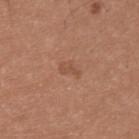No biopsy was performed on this lesion — it was imaged during a full skin examination and was not determined to be concerning.
An algorithmic analysis of the crop reported a footprint of about 3.5 mm², an outline eccentricity of about 0.8 (0 = round, 1 = elongated), and a shape-asymmetry score of about 0.35 (0 = symmetric). The analysis additionally found a mean CIELAB color near L≈51 a*≈22 b*≈31, a lesion–skin lightness drop of about 6, and a lesion-to-skin contrast of about 4.5 (normalized; higher = more distinct). It also reported a border-irregularity index near 3.5/10, internal color variation of about 0.5 on a 0–10 scale, and peripheral color asymmetry of about 0. It also reported a lesion-detection confidence of about 100/100.
Cropped from a total-body skin-imaging series; the visible field is about 15 mm.
A male subject, in their mid- to late 20s.
From the upper back.